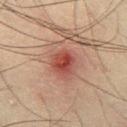This lesion was catalogued during total-body skin photography and was not selected for biopsy.
A lesion tile, about 15 mm wide, cut from a 3D total-body photograph.
A male patient, about 35 years old.
The lesion is located on the leg.